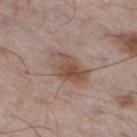Q: Was this lesion biopsied?
A: imaged on a skin check; not biopsied
Q: What kind of image is this?
A: total-body-photography crop, ~15 mm field of view
Q: How large is the lesion?
A: ~5 mm (longest diameter)
Q: Illumination type?
A: white-light illumination
Q: Patient demographics?
A: male, aged around 60
Q: Lesion location?
A: the leg